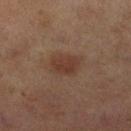A lesion tile, about 15 mm wide, cut from a 3D total-body photograph. Located on the right lower leg. This is a cross-polarized tile. Automated tile analysis of the lesion measured an area of roughly 7 mm², an eccentricity of roughly 0.7, and two-axis asymmetry of about 0.2. It also reported a lesion–skin lightness drop of about 7 and a normalized border contrast of about 6.5. The analysis additionally found border irregularity of about 2 on a 0–10 scale and internal color variation of about 1.5 on a 0–10 scale. The software also gave a nevus-likeness score of about 90/100 and a detector confidence of about 100 out of 100 that the crop contains a lesion. The patient is a female roughly 60 years of age.